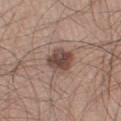| field | value |
|---|---|
| notes | no biopsy performed (imaged during a skin exam) |
| lighting | white-light illumination |
| anatomic site | the leg |
| subject | male, about 30 years old |
| TBP lesion metrics | a lesion color around L≈45 a*≈18 b*≈23 in CIELAB and a lesion-to-skin contrast of about 10 (normalized; higher = more distinct); a border-irregularity index near 2.5/10, a color-variation rating of about 4.5/10, and radial color variation of about 1.5 |
| acquisition | ~15 mm tile from a whole-body skin photo |
| diameter | ≈3.5 mm |